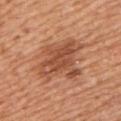{"biopsy_status": "not biopsied; imaged during a skin examination", "site": "left upper arm", "patient": {"sex": "male", "age_approx": 55}, "image": {"source": "total-body photography crop", "field_of_view_mm": 15}, "lesion_size": {"long_diameter_mm_approx": 6.0}, "automated_metrics": {"nevus_likeness_0_100": 75}}